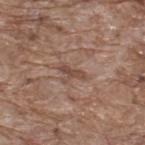A male patient aged approximately 70. A close-up tile cropped from a whole-body skin photograph, about 15 mm across. The tile uses white-light illumination. On the upper back. An algorithmic analysis of the crop reported a footprint of about 3 mm², an eccentricity of roughly 0.95, and a shape-asymmetry score of about 0.4 (0 = symmetric). The analysis additionally found a border-irregularity rating of about 4/10. The analysis additionally found a nevus-likeness score of about 0/100 and a lesion-detection confidence of about 60/100. Longest diameter approximately 3 mm.